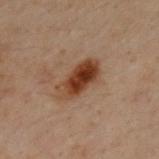workup: total-body-photography surveillance lesion; no biopsy
image-analysis metrics: a border-irregularity rating of about 2.5/10, a within-lesion color-variation index near 7.5/10, and a peripheral color-asymmetry measure near 2.5; lesion-presence confidence of about 100/100
tile lighting: cross-polarized
subject: male, roughly 30 years of age
image source: ~15 mm tile from a whole-body skin photo
body site: the upper back
size: about 5.5 mm